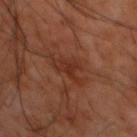– notes: no biopsy performed (imaged during a skin exam)